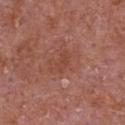{"lighting": "white-light", "automated_metrics": {"cielab_L": 44, "cielab_a": 26, "cielab_b": 29, "vs_skin_darker_L": 5.0, "vs_skin_contrast_norm": 5.0, "nevus_likeness_0_100": 0, "lesion_detection_confidence_0_100": 100}, "patient": {"sex": "male", "age_approx": 65}, "image": {"source": "total-body photography crop", "field_of_view_mm": 15}, "site": "chest"}